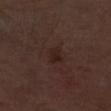About 2 mm across.
A 15 mm crop from a total-body photograph taken for skin-cancer surveillance.
On the right lower leg.
A male patient, approximately 65 years of age.
This is a white-light tile.
The lesion was biopsied, and histopathology showed a nodular basal cell carcinoma.